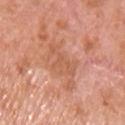Captured during whole-body skin photography for melanoma surveillance; the lesion was not biopsied. The subject is a male aged approximately 70. A region of skin cropped from a whole-body photographic capture, roughly 15 mm wide. Captured under white-light illumination. The lesion-visualizer software estimated a lesion–skin lightness drop of about 6 and a normalized lesion–skin contrast near 4.5. The analysis additionally found a within-lesion color-variation index near 1.5/10. Approximately 4 mm at its widest. On the chest.